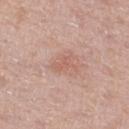The lesion was photographed on a routine skin check and not biopsied; there is no pathology result.
On the right thigh.
A female patient aged 58 to 62.
Cropped from a total-body skin-imaging series; the visible field is about 15 mm.